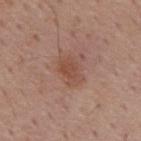biopsy status: total-body-photography surveillance lesion; no biopsy
size: about 3.5 mm
image: ~15 mm crop, total-body skin-cancer survey
subject: male, about 60 years old
automated lesion analysis: a lesion color around L≈49 a*≈21 b*≈28 in CIELAB and a normalized border contrast of about 6; a border-irregularity rating of about 3/10 and internal color variation of about 2.5 on a 0–10 scale
body site: the mid back
tile lighting: white-light